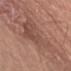Clinical impression: The lesion was photographed on a routine skin check and not biopsied; there is no pathology result.